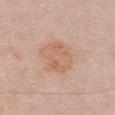workup = catalogued during a skin exam; not biopsied
body site = the chest
subject = female, aged 28–32
lesion size = ≈4.5 mm
image = total-body-photography crop, ~15 mm field of view
automated lesion analysis = a footprint of about 15 mm² and a shape eccentricity near 0.5; border irregularity of about 2 on a 0–10 scale, a color-variation rating of about 3.5/10, and radial color variation of about 1
tile lighting = white-light